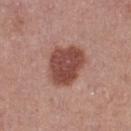Q: Is there a histopathology result?
A: total-body-photography surveillance lesion; no biopsy
Q: What are the patient's age and sex?
A: female, aged 38 to 42
Q: Lesion location?
A: the leg
Q: How was this image acquired?
A: ~15 mm tile from a whole-body skin photo